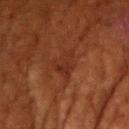On the arm. Approximately 3 mm at its widest. Captured under cross-polarized illumination. This image is a 15 mm lesion crop taken from a total-body photograph. A female patient, approximately 80 years of age.